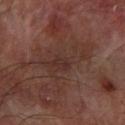Q: What is the imaging modality?
A: ~15 mm tile from a whole-body skin photo
Q: Patient demographics?
A: male, aged 63–67
Q: Where on the body is the lesion?
A: the arm
Q: Automated lesion metrics?
A: a border-irregularity rating of about 8.5/10, a color-variation rating of about 2.5/10, and peripheral color asymmetry of about 1; a classifier nevus-likeness of about 0/100
Q: How was the tile lit?
A: cross-polarized
Q: What is the lesion's diameter?
A: ≈6.5 mm